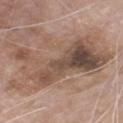The lesion was photographed on a routine skin check and not biopsied; there is no pathology result. Cropped from a total-body skin-imaging series; the visible field is about 15 mm. The tile uses white-light illumination. The lesion is located on the front of the torso. About 11.5 mm across. The patient is a male roughly 75 years of age.